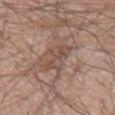Q: What kind of image is this?
A: total-body-photography crop, ~15 mm field of view
Q: What are the patient's age and sex?
A: male, about 60 years old
Q: How large is the lesion?
A: ≈3.5 mm
Q: Where on the body is the lesion?
A: the right upper arm
Q: What did automated image analysis measure?
A: a lesion color around L≈49 a*≈17 b*≈24 in CIELAB, a lesion–skin lightness drop of about 7, and a lesion-to-skin contrast of about 5.5 (normalized; higher = more distinct); a classifier nevus-likeness of about 0/100 and lesion-presence confidence of about 65/100
Q: Illumination type?
A: white-light illumination